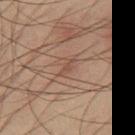Notes:
* anatomic site · the leg
* image source · total-body-photography crop, ~15 mm field of view
* subject · male, in their mid- to late 30s
* TBP lesion metrics · a lesion area of about 2.5 mm², a shape eccentricity near 0.9, and a shape-asymmetry score of about 0.3 (0 = symmetric); border irregularity of about 3.5 on a 0–10 scale, a color-variation rating of about 0/10, and peripheral color asymmetry of about 0